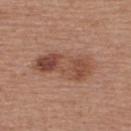No biopsy was performed on this lesion — it was imaged during a full skin examination and was not determined to be concerning. The subject is a male aged around 55. Measured at roughly 7 mm in maximum diameter. The lesion is located on the upper back. The total-body-photography lesion software estimated an area of roughly 18 mm², a shape eccentricity near 0.9, and a shape-asymmetry score of about 0.25 (0 = symmetric). The software also gave an average lesion color of about L≈48 a*≈22 b*≈29 (CIELAB). The software also gave peripheral color asymmetry of about 2.5. And it measured a classifier nevus-likeness of about 60/100. A lesion tile, about 15 mm wide, cut from a 3D total-body photograph. The tile uses white-light illumination.